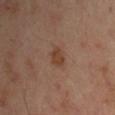Captured during whole-body skin photography for melanoma surveillance; the lesion was not biopsied. An algorithmic analysis of the crop reported a lesion color around L≈40 a*≈19 b*≈28 in CIELAB and a normalized lesion–skin contrast near 7. It also reported a color-variation rating of about 2/10 and radial color variation of about 0.5. The software also gave a nevus-likeness score of about 65/100 and a detector confidence of about 100 out of 100 that the crop contains a lesion. A male patient, aged 48–52. The lesion's longest dimension is about 2.5 mm. Cropped from a whole-body photographic skin survey; the tile spans about 15 mm. The tile uses cross-polarized illumination. Located on the left upper arm.